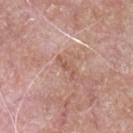Impression: No biopsy was performed on this lesion — it was imaged during a full skin examination and was not determined to be concerning. Context: The lesion is located on the chest. A male patient, aged around 65. A 15 mm close-up tile from a total-body photography series done for melanoma screening.